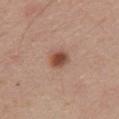Captured during whole-body skin photography for melanoma surveillance; the lesion was not biopsied.